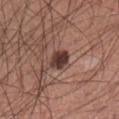Q: Is there a histopathology result?
A: no biopsy performed (imaged during a skin exam)
Q: Lesion size?
A: about 2.5 mm
Q: Where on the body is the lesion?
A: the right lower leg
Q: What is the imaging modality?
A: ~15 mm tile from a whole-body skin photo
Q: Illumination type?
A: white-light illumination
Q: Patient demographics?
A: male, in their mid- to late 40s
Q: What did automated image analysis measure?
A: about 14 CIELAB-L* units darker than the surrounding skin and a lesion-to-skin contrast of about 12 (normalized; higher = more distinct); a border-irregularity rating of about 2/10, internal color variation of about 4 on a 0–10 scale, and peripheral color asymmetry of about 1.5; a detector confidence of about 100 out of 100 that the crop contains a lesion